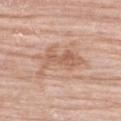No biopsy was performed on this lesion — it was imaged during a full skin examination and was not determined to be concerning.
An algorithmic analysis of the crop reported an area of roughly 12 mm². And it measured a lesion–skin lightness drop of about 9 and a normalized lesion–skin contrast near 6.5. And it measured an automated nevus-likeness rating near 0 out of 100 and lesion-presence confidence of about 95/100.
A region of skin cropped from a whole-body photographic capture, roughly 15 mm wide.
A female subject, about 70 years old.
This is a white-light tile.
The lesion is on the upper back.